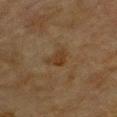Case summary:
– workup — catalogued during a skin exam; not biopsied
– site — the front of the torso
– lighting — cross-polarized illumination
– size — ≈3 mm
– patient — male, approximately 85 years of age or older
– image — 15 mm crop, total-body photography
– image-analysis metrics — a lesion color around L≈31 a*≈15 b*≈28 in CIELAB and a lesion–skin lightness drop of about 6; a border-irregularity index near 3.5/10, internal color variation of about 2 on a 0–10 scale, and a peripheral color-asymmetry measure near 0.5